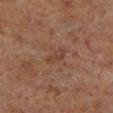| field | value |
|---|---|
| notes | catalogued during a skin exam; not biopsied |
| diameter | ~3 mm (longest diameter) |
| acquisition | total-body-photography crop, ~15 mm field of view |
| automated metrics | a border-irregularity index near 3.5/10, internal color variation of about 2 on a 0–10 scale, and a peripheral color-asymmetry measure near 1; a nevus-likeness score of about 0/100 and a detector confidence of about 100 out of 100 that the crop contains a lesion |
| subject | male, about 60 years old |
| location | the left lower leg |